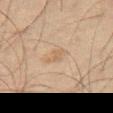Part of a total-body skin-imaging series; this lesion was reviewed on a skin check and was not flagged for biopsy. This is a cross-polarized tile. The recorded lesion diameter is about 3 mm. A 15 mm close-up tile from a total-body photography series done for melanoma screening. A male patient aged around 65. Located on the right thigh.